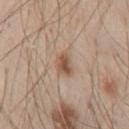Part of a total-body skin-imaging series; this lesion was reviewed on a skin check and was not flagged for biopsy. A male patient in their mid- to late 40s. On the chest. Measured at roughly 3 mm in maximum diameter. The total-body-photography lesion software estimated a mean CIELAB color near L≈54 a*≈17 b*≈29, a lesion–skin lightness drop of about 11, and a normalized border contrast of about 8. The software also gave border irregularity of about 2 on a 0–10 scale, a within-lesion color-variation index near 4/10, and radial color variation of about 1.5. And it measured an automated nevus-likeness rating near 85 out of 100 and a detector confidence of about 100 out of 100 that the crop contains a lesion. A roughly 15 mm field-of-view crop from a total-body skin photograph. Imaged with white-light lighting.